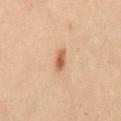<lesion>
  <biopsy_status>not biopsied; imaged during a skin examination</biopsy_status>
  <image>
    <source>total-body photography crop</source>
    <field_of_view_mm>15</field_of_view_mm>
  </image>
  <patient>
    <sex>female</sex>
    <age_approx>30</age_approx>
  </patient>
  <site>lower back</site>
  <lighting>cross-polarized</lighting>
  <lesion_size>
    <long_diameter_mm_approx>2.5</long_diameter_mm_approx>
  </lesion_size>
</lesion>